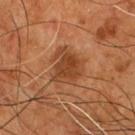Case summary:
• biopsy status · no biopsy performed (imaged during a skin exam)
• acquisition · ~15 mm crop, total-body skin-cancer survey
• lesion size · about 4 mm
• subject · male, aged 53 to 57
• body site · the front of the torso